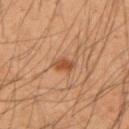Part of a total-body skin-imaging series; this lesion was reviewed on a skin check and was not flagged for biopsy.
This image is a 15 mm lesion crop taken from a total-body photograph.
Imaged with cross-polarized lighting.
The lesion is on the left forearm.
The patient is a male about 35 years old.
The lesion-visualizer software estimated a footprint of about 3.5 mm² and a shape eccentricity near 0.8. It also reported border irregularity of about 3 on a 0–10 scale and peripheral color asymmetry of about 0.5.